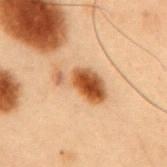Q: Was a biopsy performed?
A: no biopsy performed (imaged during a skin exam)
Q: What is the imaging modality?
A: ~15 mm crop, total-body skin-cancer survey
Q: Who is the patient?
A: male, aged approximately 55
Q: Where on the body is the lesion?
A: the back